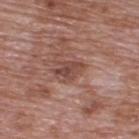Q: Was this lesion biopsied?
A: no biopsy performed (imaged during a skin exam)
Q: Patient demographics?
A: male, roughly 70 years of age
Q: Automated lesion metrics?
A: an area of roughly 5 mm², an eccentricity of roughly 0.75, and two-axis asymmetry of about 0.3; a lesion color around L≈45 a*≈22 b*≈24 in CIELAB, a lesion–skin lightness drop of about 9, and a normalized lesion–skin contrast near 7; a within-lesion color-variation index near 3/10 and a peripheral color-asymmetry measure near 1
Q: What is the anatomic site?
A: the upper back
Q: Illumination type?
A: white-light illumination
Q: What kind of image is this?
A: total-body-photography crop, ~15 mm field of view